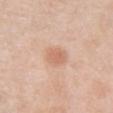Clinical impression: This lesion was catalogued during total-body skin photography and was not selected for biopsy. Background: Cropped from a whole-body photographic skin survey; the tile spans about 15 mm. A female subject aged around 45. The lesion is on the chest.